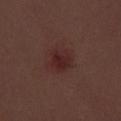Clinical impression: Part of a total-body skin-imaging series; this lesion was reviewed on a skin check and was not flagged for biopsy. Acquisition and patient details: Cropped from a whole-body photographic skin survey; the tile spans about 15 mm. Measured at roughly 3 mm in maximum diameter. The subject is a female about 30 years old. On the left thigh. Imaged with white-light lighting.